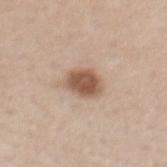follow-up: total-body-photography surveillance lesion; no biopsy | body site: the mid back | imaging modality: 15 mm crop, total-body photography | patient: female, aged around 45 | size: ~4 mm (longest diameter) | TBP lesion metrics: an average lesion color of about L≈55 a*≈18 b*≈29 (CIELAB), about 15 CIELAB-L* units darker than the surrounding skin, and a lesion-to-skin contrast of about 9.5 (normalized; higher = more distinct); an automated nevus-likeness rating near 95 out of 100 and lesion-presence confidence of about 100/100 | illumination: white-light.